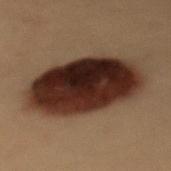The lesion was tiled from a total-body skin photograph and was not biopsied.
A region of skin cropped from a whole-body photographic capture, roughly 15 mm wide.
A female subject in their 40s.
Located on the mid back.
Imaged with cross-polarized lighting.
Measured at roughly 10.5 mm in maximum diameter.